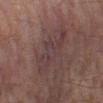Captured during whole-body skin photography for melanoma surveillance; the lesion was not biopsied.
The recorded lesion diameter is about 7 mm.
Cropped from a total-body skin-imaging series; the visible field is about 15 mm.
A male patient aged 63–67.
Located on the leg.